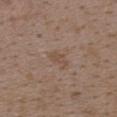imaging modality — total-body-photography crop, ~15 mm field of view
location — the back
subject — female, aged around 35
lesion diameter — about 2.5 mm
tile lighting — white-light illumination
automated lesion analysis — a lesion area of about 2.5 mm², an outline eccentricity of about 0.85 (0 = round, 1 = elongated), and two-axis asymmetry of about 0.35; a lesion color around L≈48 a*≈16 b*≈27 in CIELAB and roughly 6 lightness units darker than nearby skin